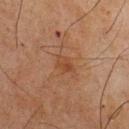Impression:
Recorded during total-body skin imaging; not selected for excision or biopsy.
Acquisition and patient details:
A male subject, roughly 75 years of age. A close-up tile cropped from a whole-body skin photograph, about 15 mm across. From the chest. Automated image analysis of the tile measured a peripheral color-asymmetry measure near 1. The software also gave a lesion-detection confidence of about 100/100. The lesion's longest dimension is about 2.5 mm.